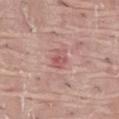biopsy status: total-body-photography surveillance lesion; no biopsy
TBP lesion metrics: a border-irregularity rating of about 2/10 and a peripheral color-asymmetry measure near 2
diameter: ≈2.5 mm
tile lighting: white-light illumination
anatomic site: the abdomen
subject: male, aged approximately 40
imaging modality: total-body-photography crop, ~15 mm field of view